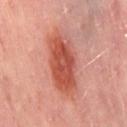The lesion was photographed on a routine skin check and not biopsied; there is no pathology result.
From the abdomen.
A female patient aged 48 to 52.
A roughly 15 mm field-of-view crop from a total-body skin photograph.